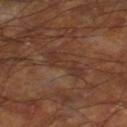Clinical impression: The lesion was tiled from a total-body skin photograph and was not biopsied. Image and clinical context: Located on the right lower leg. A male patient, aged around 60. The tile uses cross-polarized illumination. Automated image analysis of the tile measured a mean CIELAB color near L≈32 a*≈19 b*≈25. It also reported a border-irregularity index near 5.5/10, a color-variation rating of about 2.5/10, and radial color variation of about 0.5. The analysis additionally found lesion-presence confidence of about 80/100. Approximately 5 mm at its widest. A lesion tile, about 15 mm wide, cut from a 3D total-body photograph.